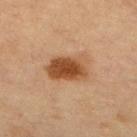notes — imaged on a skin check; not biopsied